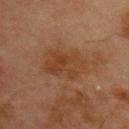Imaged with cross-polarized lighting. The lesion-visualizer software estimated a lesion area of about 15 mm². And it measured border irregularity of about 2.5 on a 0–10 scale, a within-lesion color-variation index near 3.5/10, and radial color variation of about 1. The lesion is on the chest. Measured at roughly 5 mm in maximum diameter. A male patient, aged 68 to 72. A close-up tile cropped from a whole-body skin photograph, about 15 mm across.